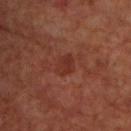The lesion was photographed on a routine skin check and not biopsied; there is no pathology result. A male subject about 65 years old. From the chest. Cropped from a whole-body photographic skin survey; the tile spans about 15 mm.